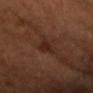notes: total-body-photography surveillance lesion; no biopsy
body site: the head or neck
subject: male, roughly 40 years of age
lighting: cross-polarized
image: total-body-photography crop, ~15 mm field of view
automated lesion analysis: an average lesion color of about L≈25 a*≈20 b*≈26 (CIELAB), a lesion–skin lightness drop of about 7, and a lesion-to-skin contrast of about 7.5 (normalized; higher = more distinct); a border-irregularity rating of about 4/10 and a within-lesion color-variation index near 1/10; lesion-presence confidence of about 95/100